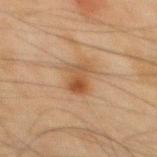Imaged during a routine full-body skin examination; the lesion was not biopsied and no histopathology is available. Captured under cross-polarized illumination. A male patient in their mid- to late 40s. About 4 mm across. A roughly 15 mm field-of-view crop from a total-body skin photograph. On the mid back.